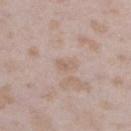| feature | finding |
|---|---|
| biopsy status | total-body-photography surveillance lesion; no biopsy |
| illumination | white-light illumination |
| diameter | ≈2.5 mm |
| automated lesion analysis | a lesion area of about 3 mm², an eccentricity of roughly 0.8, and two-axis asymmetry of about 0.4; about 6 CIELAB-L* units darker than the surrounding skin and a normalized border contrast of about 4.5 |
| image source | ~15 mm crop, total-body skin-cancer survey |
| subject | female, in their mid-20s |
| site | the left lower leg |